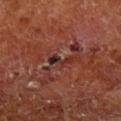The lesion was tiled from a total-body skin photograph and was not biopsied. Cropped from a total-body skin-imaging series; the visible field is about 15 mm. A male subject roughly 65 years of age. Approximately 3 mm at its widest. The total-body-photography lesion software estimated a footprint of about 3 mm², an outline eccentricity of about 0.9 (0 = round, 1 = elongated), and a shape-asymmetry score of about 0.6 (0 = symmetric). The software also gave a border-irregularity rating of about 7.5/10 and a within-lesion color-variation index near 0/10. This is a cross-polarized tile. On the left lower leg.